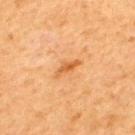size = ≈3 mm
lighting = cross-polarized illumination
image-analysis metrics = a lesion area of about 3 mm², a shape eccentricity near 0.95, and two-axis asymmetry of about 0.45; internal color variation of about 0.5 on a 0–10 scale and a peripheral color-asymmetry measure near 0.5; an automated nevus-likeness rating near 30 out of 100
location = the upper back
image source = ~15 mm tile from a whole-body skin photo
subject = male, roughly 65 years of age